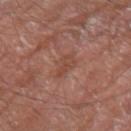The lesion-visualizer software estimated an area of roughly 3.5 mm², a shape eccentricity near 0.85, and a symmetry-axis asymmetry near 0.35. The analysis additionally found a lesion color around L≈46 a*≈23 b*≈28 in CIELAB, a lesion–skin lightness drop of about 7, and a normalized lesion–skin contrast near 5.5. The analysis additionally found an automated nevus-likeness rating near 0 out of 100 and lesion-presence confidence of about 100/100. Imaged with white-light lighting. Cropped from a total-body skin-imaging series; the visible field is about 15 mm. The lesion is located on the right forearm. Longest diameter approximately 3 mm. The subject is a male aged 78–82.